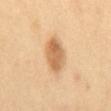Assessment: Imaged during a routine full-body skin examination; the lesion was not biopsied and no histopathology is available. Acquisition and patient details: From the mid back. A female patient, aged 58–62. A 15 mm crop from a total-body photograph taken for skin-cancer surveillance.